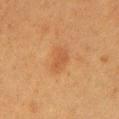Assessment: Part of a total-body skin-imaging series; this lesion was reviewed on a skin check and was not flagged for biopsy. Image and clinical context: The lesion is located on the left upper arm. A female subject, aged 38–42. A lesion tile, about 15 mm wide, cut from a 3D total-body photograph.